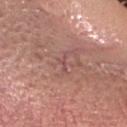Captured during whole-body skin photography for melanoma surveillance; the lesion was not biopsied. From the head or neck. Cropped from a whole-body photographic skin survey; the tile spans about 15 mm. A male subject aged 63 to 67.